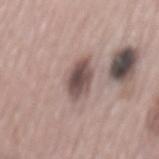Recorded during total-body skin imaging; not selected for excision or biopsy.